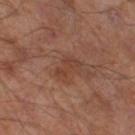No biopsy was performed on this lesion — it was imaged during a full skin examination and was not determined to be concerning.
Automated tile analysis of the lesion measured an automated nevus-likeness rating near 0 out of 100 and a lesion-detection confidence of about 100/100.
From the right thigh.
Approximately 3 mm at its widest.
A 15 mm close-up tile from a total-body photography series done for melanoma screening.
The subject is a male in their mid- to late 60s.